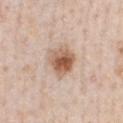workup=imaged on a skin check; not biopsied | size=about 3.5 mm | illumination=white-light | image source=~15 mm tile from a whole-body skin photo | site=the chest | patient=male, aged around 60.